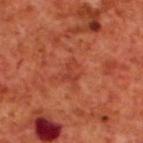| key | value |
|---|---|
| tile lighting | cross-polarized |
| anatomic site | the upper back |
| patient | male, about 70 years old |
| image source | total-body-photography crop, ~15 mm field of view |
| automated metrics | a footprint of about 3 mm² and a shape eccentricity near 0.8; border irregularity of about 5 on a 0–10 scale, a color-variation rating of about 0/10, and radial color variation of about 0 |
| diameter | about 2.5 mm |